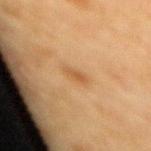Acquisition and patient details: The lesion is located on the mid back. The subject is a female aged approximately 55. A roughly 15 mm field-of-view crop from a total-body skin photograph.